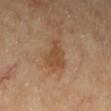Notes:
• biopsy status — catalogued during a skin exam; not biopsied
• subject — female, aged 58 to 62
• anatomic site — the left lower leg
• tile lighting — cross-polarized illumination
• diameter — about 4 mm
• image — ~15 mm crop, total-body skin-cancer survey